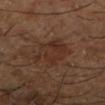notes=imaged on a skin check; not biopsied | anatomic site=the left lower leg | lighting=cross-polarized illumination | image=total-body-photography crop, ~15 mm field of view | patient=male, in their mid- to late 60s | diameter=~5 mm (longest diameter).